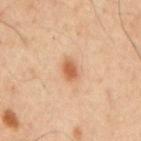The lesion was tiled from a total-body skin photograph and was not biopsied.